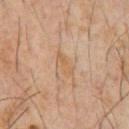The lesion is located on the chest. A male subject approximately 60 years of age. Automated image analysis of the tile measured an eccentricity of roughly 0.85. The software also gave a classifier nevus-likeness of about 0/100 and a detector confidence of about 100 out of 100 that the crop contains a lesion. A region of skin cropped from a whole-body photographic capture, roughly 15 mm wide.